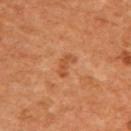Case summary:
– follow-up · no biopsy performed (imaged during a skin exam)
– lesion diameter · about 3 mm
– tile lighting · cross-polarized
– image source · ~15 mm tile from a whole-body skin photo
– patient · male, aged 58 to 62
– anatomic site · the back